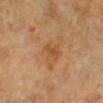Impression: Imaged during a routine full-body skin examination; the lesion was not biopsied and no histopathology is available. Acquisition and patient details: The subject is a female approximately 55 years of age. The lesion is located on the right lower leg. A roughly 15 mm field-of-view crop from a total-body skin photograph.